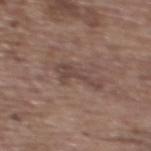Q: Is there a histopathology result?
A: no biopsy performed (imaged during a skin exam)
Q: What is the imaging modality?
A: total-body-photography crop, ~15 mm field of view
Q: Who is the patient?
A: female, in their mid- to late 60s
Q: How was the tile lit?
A: white-light
Q: What is the anatomic site?
A: the upper back
Q: Automated lesion metrics?
A: a footprint of about 5.5 mm² and a shape-asymmetry score of about 0.65 (0 = symmetric); an average lesion color of about L≈44 a*≈16 b*≈21 (CIELAB), roughly 7 lightness units darker than nearby skin, and a normalized lesion–skin contrast near 6